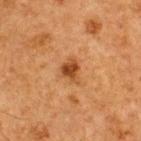Clinical summary: The lesion is located on the upper back. A 15 mm close-up tile from a total-body photography series done for melanoma screening. A male subject, aged approximately 65.